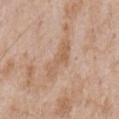Notes:
• follow-up · imaged on a skin check; not biopsied
• image · ~15 mm crop, total-body skin-cancer survey
• subject · male, in their mid-60s
• site · the chest
• automated lesion analysis · an area of roughly 7 mm², an eccentricity of roughly 0.95, and two-axis asymmetry of about 0.4; a nevus-likeness score of about 0/100 and a lesion-detection confidence of about 85/100
• size · ≈5 mm
• tile lighting · white-light